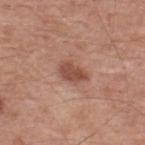The lesion was tiled from a total-body skin photograph and was not biopsied.
Longest diameter approximately 3.5 mm.
This is a white-light tile.
The lesion is on the back.
A male subject, roughly 55 years of age.
A region of skin cropped from a whole-body photographic capture, roughly 15 mm wide.